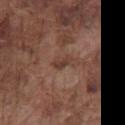biopsy status: imaged on a skin check; not biopsied
patient: male, aged 73 to 77
body site: the front of the torso
image-analysis metrics: a footprint of about 3.5 mm², a shape eccentricity near 0.8, and a symmetry-axis asymmetry near 0.25; a lesion color around L≈39 a*≈19 b*≈24 in CIELAB, a lesion–skin lightness drop of about 7, and a lesion-to-skin contrast of about 6.5 (normalized; higher = more distinct)
lighting: white-light
size: ≈2.5 mm
imaging modality: 15 mm crop, total-body photography